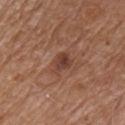  biopsy_status: not biopsied; imaged during a skin examination
  patient:
    sex: male
    age_approx: 80
  lighting: white-light
  automated_metrics:
    cielab_L: 41
    cielab_a: 21
    cielab_b: 27
    vs_skin_darker_L: 9.0
    border_irregularity_0_10: 2.5
    color_variation_0_10: 3.5
    peripheral_color_asymmetry: 1.5
    nevus_likeness_0_100: 10
    lesion_detection_confidence_0_100: 100
  image:
    source: total-body photography crop
    field_of_view_mm: 15
  lesion_size:
    long_diameter_mm_approx: 3.0
  site: front of the torso